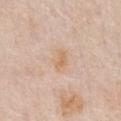On the front of the torso.
A 15 mm crop from a total-body photograph taken for skin-cancer surveillance.
The lesion-visualizer software estimated a mean CIELAB color near L≈68 a*≈17 b*≈34 and a normalized lesion–skin contrast near 6.
The patient is a male in their mid-70s.